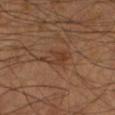{"biopsy_status": "not biopsied; imaged during a skin examination", "site": "left lower leg", "patient": {"sex": "male", "age_approx": 55}, "lighting": "cross-polarized", "lesion_size": {"long_diameter_mm_approx": 4.0}, "image": {"source": "total-body photography crop", "field_of_view_mm": 15}, "automated_metrics": {"cielab_L": 34, "cielab_a": 18, "cielab_b": 27, "vs_skin_darker_L": 6.0, "vs_skin_contrast_norm": 6.0, "border_irregularity_0_10": 4.5, "color_variation_0_10": 3.5, "peripheral_color_asymmetry": 1.0}}